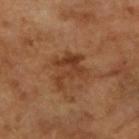biopsy status: no biopsy performed (imaged during a skin exam) | illumination: cross-polarized | body site: the arm | imaging modality: ~15 mm tile from a whole-body skin photo | subject: female, approximately 70 years of age.